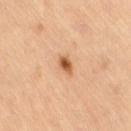Q: Was a biopsy performed?
A: total-body-photography surveillance lesion; no biopsy
Q: Lesion location?
A: the leg
Q: How was the tile lit?
A: cross-polarized illumination
Q: What are the patient's age and sex?
A: female, aged 68 to 72
Q: Lesion size?
A: ≈2.5 mm
Q: What is the imaging modality?
A: 15 mm crop, total-body photography
Q: What did automated image analysis measure?
A: an area of roughly 3.5 mm² and a symmetry-axis asymmetry near 0.2; an average lesion color of about L≈60 a*≈25 b*≈40 (CIELAB) and a lesion–skin lightness drop of about 15; internal color variation of about 5 on a 0–10 scale